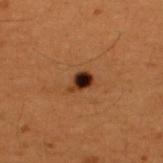notes — no biopsy performed (imaged during a skin exam) | body site — the upper back | image — ~15 mm tile from a whole-body skin photo | automated metrics — a lesion area of about 4 mm² and a shape-asymmetry score of about 0.3 (0 = symmetric); a lesion color around L≈22 a*≈19 b*≈23 in CIELAB, a lesion–skin lightness drop of about 15, and a normalized border contrast of about 16.5 | lighting — cross-polarized | patient — male, aged around 50 | lesion diameter — about 2.5 mm.